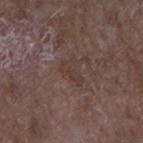follow-up — imaged on a skin check; not biopsied
imaging modality — ~15 mm crop, total-body skin-cancer survey
subject — male, in their mid-60s
body site — the left forearm